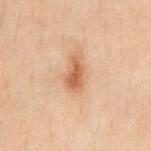This lesion was catalogued during total-body skin photography and was not selected for biopsy. The lesion is on the abdomen. The total-body-photography lesion software estimated a mean CIELAB color near L≈49 a*≈19 b*≈31. It also reported a border-irregularity index near 3.5/10, a color-variation rating of about 2.5/10, and radial color variation of about 1. It also reported a nevus-likeness score of about 95/100 and a detector confidence of about 100 out of 100 that the crop contains a lesion. This is a cross-polarized tile. A male patient aged around 30. A 15 mm close-up extracted from a 3D total-body photography capture. Measured at roughly 3.5 mm in maximum diameter.